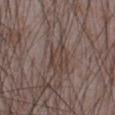The lesion was tiled from a total-body skin photograph and was not biopsied.
A 15 mm close-up tile from a total-body photography series done for melanoma screening.
A male patient aged approximately 75.
The lesion is on the front of the torso.
The recorded lesion diameter is about 3 mm.
Captured under white-light illumination.